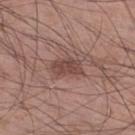tile lighting: white-light; diameter: about 4 mm; subject: male, in their 60s; anatomic site: the right lower leg; acquisition: 15 mm crop, total-body photography.